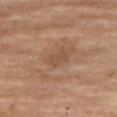Acquisition and patient details:
The subject is a female aged 68–72. A 15 mm crop from a total-body photograph taken for skin-cancer surveillance. The lesion is on the right thigh.